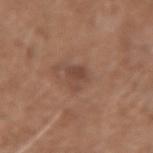workup: total-body-photography surveillance lesion; no biopsy
automated lesion analysis: an area of roughly 4.5 mm², an eccentricity of roughly 0.7, and a symmetry-axis asymmetry near 0.4; a border-irregularity rating of about 4/10, internal color variation of about 1.5 on a 0–10 scale, and a peripheral color-asymmetry measure near 0.5; a nevus-likeness score of about 15/100 and a detector confidence of about 100 out of 100 that the crop contains a lesion
lesion diameter: ≈2.5 mm
patient: female, aged 38–42
imaging modality: ~15 mm crop, total-body skin-cancer survey
illumination: white-light illumination
anatomic site: the left forearm